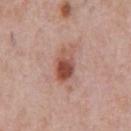<case>
<biopsy_status>not biopsied; imaged during a skin examination</biopsy_status>
<image>
  <source>total-body photography crop</source>
  <field_of_view_mm>15</field_of_view_mm>
</image>
<patient>
  <sex>male</sex>
  <age_approx>40</age_approx>
</patient>
<site>chest</site>
</case>